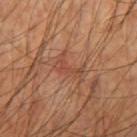This lesion was catalogued during total-body skin photography and was not selected for biopsy. On the right upper arm. The recorded lesion diameter is about 3.5 mm. A roughly 15 mm field-of-view crop from a total-body skin photograph. A male subject, aged approximately 60. Captured under cross-polarized illumination. Automated image analysis of the tile measured a lesion area of about 4.5 mm², a shape eccentricity near 0.85, and two-axis asymmetry of about 0.55. The analysis additionally found a border-irregularity rating of about 6.5/10, a color-variation rating of about 1/10, and peripheral color asymmetry of about 0.5. It also reported a detector confidence of about 90 out of 100 that the crop contains a lesion.